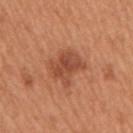{"biopsy_status": "not biopsied; imaged during a skin examination", "image": {"source": "total-body photography crop", "field_of_view_mm": 15}, "site": "right upper arm", "patient": {"sex": "male", "age_approx": 65}, "lighting": "white-light", "lesion_size": {"long_diameter_mm_approx": 4.0}}